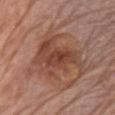notes=no biopsy performed (imaged during a skin exam)
patient=male, aged 78 to 82
size=≈7 mm
body site=the chest
imaging modality=~15 mm crop, total-body skin-cancer survey
automated metrics=about 9 CIELAB-L* units darker than the surrounding skin and a normalized lesion–skin contrast near 7.5; a within-lesion color-variation index near 5.5/10 and a peripheral color-asymmetry measure near 2; a classifier nevus-likeness of about 30/100